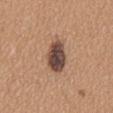Image and clinical context:
On the back. Automated tile analysis of the lesion measured a border-irregularity rating of about 2.5/10 and a peripheral color-asymmetry measure near 1.5. Captured under white-light illumination. The subject is a female about 45 years old. Approximately 5 mm at its widest. A close-up tile cropped from a whole-body skin photograph, about 15 mm across.